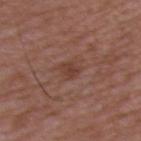| field | value |
|---|---|
| notes | total-body-photography surveillance lesion; no biopsy |
| location | the upper back |
| image-analysis metrics | an area of roughly 3.5 mm², an outline eccentricity of about 0.8 (0 = round, 1 = elongated), and two-axis asymmetry of about 0.3; a border-irregularity index near 2.5/10, a within-lesion color-variation index near 1.5/10, and radial color variation of about 0.5 |
| lesion diameter | ≈2.5 mm |
| imaging modality | ~15 mm crop, total-body skin-cancer survey |
| patient | male, about 50 years old |